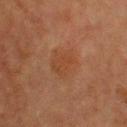Case summary:
• subject — male, roughly 60 years of age
• location — the upper back
• tile lighting — cross-polarized
• lesion size — ~3.5 mm (longest diameter)
• acquisition — ~15 mm crop, total-body skin-cancer survey